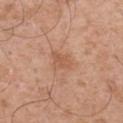The lesion was photographed on a routine skin check and not biopsied; there is no pathology result.
An algorithmic analysis of the crop reported a lesion area of about 3.5 mm² and an outline eccentricity of about 0.7 (0 = round, 1 = elongated). It also reported a mean CIELAB color near L≈56 a*≈23 b*≈33.
Longest diameter approximately 2.5 mm.
A region of skin cropped from a whole-body photographic capture, roughly 15 mm wide.
From the right upper arm.
A male subject, about 50 years old.
This is a white-light tile.